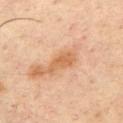Impression:
Part of a total-body skin-imaging series; this lesion was reviewed on a skin check and was not flagged for biopsy.
Acquisition and patient details:
A male subject roughly 35 years of age. This is a cross-polarized tile. Approximately 4 mm at its widest. Cropped from a whole-body photographic skin survey; the tile spans about 15 mm. The lesion is located on the front of the torso.